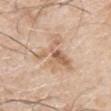Clinical impression:
No biopsy was performed on this lesion — it was imaged during a full skin examination and was not determined to be concerning.
Image and clinical context:
The recorded lesion diameter is about 6 mm. Cropped from a total-body skin-imaging series; the visible field is about 15 mm. Imaged with white-light lighting. From the arm. The subject is a male about 65 years old.